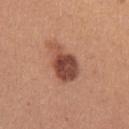Acquisition and patient details: The total-body-photography lesion software estimated a lesion area of about 12 mm² and an eccentricity of roughly 0.8. It also reported about 14 CIELAB-L* units darker than the surrounding skin and a lesion-to-skin contrast of about 10 (normalized; higher = more distinct). The software also gave a border-irregularity rating of about 3.5/10. Imaged with white-light lighting. The patient is a female in their mid- to late 20s. Located on the arm. A region of skin cropped from a whole-body photographic capture, roughly 15 mm wide.